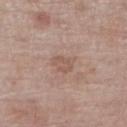No biopsy was performed on this lesion — it was imaged during a full skin examination and was not determined to be concerning. A roughly 15 mm field-of-view crop from a total-body skin photograph. The subject is a male approximately 70 years of age. The lesion's longest dimension is about 3 mm. The lesion is located on the right lower leg. Imaged with white-light lighting.